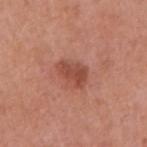• patient · male, roughly 55 years of age
• anatomic site · the arm
• automated lesion analysis · an outline eccentricity of about 0.8 (0 = round, 1 = elongated) and two-axis asymmetry of about 0.3; a classifier nevus-likeness of about 60/100 and a detector confidence of about 100 out of 100 that the crop contains a lesion
• lesion size · about 3.5 mm
• tile lighting · white-light illumination
• imaging modality · ~15 mm tile from a whole-body skin photo